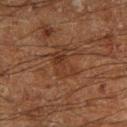workup=no biopsy performed (imaged during a skin exam); image source=~15 mm tile from a whole-body skin photo; subject=male, aged 58 to 62; anatomic site=the right lower leg.